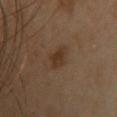The lesion was tiled from a total-body skin photograph and was not biopsied. On the upper back. This is a cross-polarized tile. A lesion tile, about 15 mm wide, cut from a 3D total-body photograph. A female patient about 40 years old. Automated tile analysis of the lesion measured a lesion color around L≈34 a*≈17 b*≈28 in CIELAB, about 7 CIELAB-L* units darker than the surrounding skin, and a normalized border contrast of about 7.5. The analysis additionally found lesion-presence confidence of about 100/100.